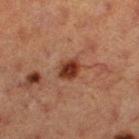The lesion was photographed on a routine skin check and not biopsied; there is no pathology result. This is a cross-polarized tile. A female patient, aged approximately 40. The lesion is on the left thigh. A 15 mm crop from a total-body photograph taken for skin-cancer surveillance. Measured at roughly 3 mm in maximum diameter.